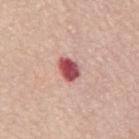{"automated_metrics": {"border_irregularity_0_10": 2.0, "color_variation_0_10": 6.5, "peripheral_color_asymmetry": 2.0, "nevus_likeness_0_100": 0, "lesion_detection_confidence_0_100": 100}, "patient": {"sex": "female", "age_approx": 70}, "lesion_size": {"long_diameter_mm_approx": 3.0}, "site": "mid back", "lighting": "white-light", "image": {"source": "total-body photography crop", "field_of_view_mm": 15}}